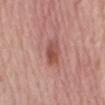notes: imaged on a skin check; not biopsied
acquisition: ~15 mm tile from a whole-body skin photo
lighting: white-light illumination
subject: female, aged 73 to 77
site: the mid back